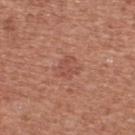Case summary:
* follow-up: total-body-photography surveillance lesion; no biopsy
* anatomic site: the chest
* lesion diameter: ~3 mm (longest diameter)
* lighting: white-light illumination
* TBP lesion metrics: a classifier nevus-likeness of about 0/100 and a lesion-detection confidence of about 100/100
* patient: male, about 45 years old
* acquisition: ~15 mm tile from a whole-body skin photo